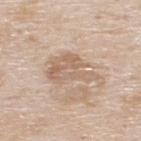workup: imaged on a skin check; not biopsied
lighting: white-light
subject: male, aged approximately 80
image source: 15 mm crop, total-body photography
automated lesion analysis: a shape eccentricity near 0.85 and a symmetry-axis asymmetry near 0.5; a nevus-likeness score of about 0/100
location: the upper back